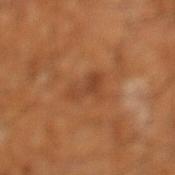biopsy_status: not biopsied; imaged during a skin examination
image:
  source: total-body photography crop
  field_of_view_mm: 15
site: left lower leg
patient:
  sex: male
  age_approx: 65